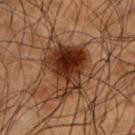Impression:
Captured during whole-body skin photography for melanoma surveillance; the lesion was not biopsied.
Context:
The lesion's longest dimension is about 7.5 mm. A region of skin cropped from a whole-body photographic capture, roughly 15 mm wide. The patient is a male approximately 50 years of age. On the left upper arm.